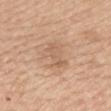Acquisition and patient details:
Automated image analysis of the tile measured a lesion area of about 8.5 mm² and an outline eccentricity of about 0.75 (0 = round, 1 = elongated). The software also gave a border-irregularity rating of about 4.5/10 and internal color variation of about 2.5 on a 0–10 scale. A female patient aged approximately 65. The lesion's longest dimension is about 4 mm. The lesion is on the mid back. A 15 mm close-up tile from a total-body photography series done for melanoma screening.